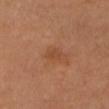<case>
  <biopsy_status>not biopsied; imaged during a skin examination</biopsy_status>
  <lighting>cross-polarized</lighting>
  <image>
    <source>total-body photography crop</source>
    <field_of_view_mm>15</field_of_view_mm>
  </image>
  <site>head or neck</site>
  <patient>
    <sex>female</sex>
    <age_approx>45</age_approx>
  </patient>
</case>